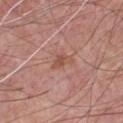Notes:
* follow-up · no biopsy performed (imaged during a skin exam)
* image · ~15 mm tile from a whole-body skin photo
* tile lighting · white-light illumination
* subject · male, about 55 years old
* anatomic site · the chest
* diameter · ~3 mm (longest diameter)